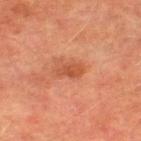This lesion was catalogued during total-body skin photography and was not selected for biopsy. A male subject aged 73–77. An algorithmic analysis of the crop reported a lesion color around L≈43 a*≈26 b*≈33 in CIELAB, a lesion–skin lightness drop of about 8, and a normalized border contrast of about 7. And it measured a within-lesion color-variation index near 1.5/10 and a peripheral color-asymmetry measure near 0.5. The analysis additionally found an automated nevus-likeness rating near 25 out of 100 and lesion-presence confidence of about 100/100. Located on the left thigh. A 15 mm close-up extracted from a 3D total-body photography capture. The recorded lesion diameter is about 2.5 mm. This is a cross-polarized tile.